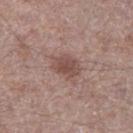{"biopsy_status": "not biopsied; imaged during a skin examination", "patient": {"sex": "male", "age_approx": 70}, "image": {"source": "total-body photography crop", "field_of_view_mm": 15}, "lesion_size": {"long_diameter_mm_approx": 3.5}, "site": "left thigh", "lighting": "white-light", "automated_metrics": {"area_mm2_approx": 6.0, "eccentricity": 0.7, "border_irregularity_0_10": 2.5}}